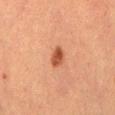No biopsy was performed on this lesion — it was imaged during a full skin examination and was not determined to be concerning. Imaged with cross-polarized lighting. From the back. A region of skin cropped from a whole-body photographic capture, roughly 15 mm wide. A male subject in their 50s.